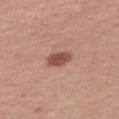Clinical summary:
A region of skin cropped from a whole-body photographic capture, roughly 15 mm wide. A female subject aged around 40. On the right thigh.